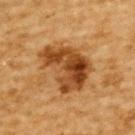Impression:
Part of a total-body skin-imaging series; this lesion was reviewed on a skin check and was not flagged for biopsy.
Context:
A male subject, approximately 85 years of age or older. On the upper back. A 15 mm close-up tile from a total-body photography series done for melanoma screening. The total-body-photography lesion software estimated a lesion area of about 19 mm², an outline eccentricity of about 0.7 (0 = round, 1 = elongated), and a symmetry-axis asymmetry near 0.45. It also reported about 13 CIELAB-L* units darker than the surrounding skin and a normalized border contrast of about 11. The software also gave border irregularity of about 5.5 on a 0–10 scale, a color-variation rating of about 8/10, and peripheral color asymmetry of about 3. It also reported an automated nevus-likeness rating near 65 out of 100 and lesion-presence confidence of about 100/100. The recorded lesion diameter is about 6 mm. This is a cross-polarized tile.